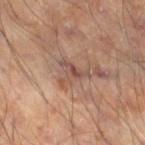Imaged during a routine full-body skin examination; the lesion was not biopsied and no histopathology is available.
About 3.5 mm across.
The lesion is located on the right thigh.
The patient is a male aged 53–57.
A close-up tile cropped from a whole-body skin photograph, about 15 mm across.
Captured under cross-polarized illumination.
An algorithmic analysis of the crop reported a mean CIELAB color near L≈46 a*≈18 b*≈24, roughly 8 lightness units darker than nearby skin, and a normalized lesion–skin contrast near 6. And it measured a border-irregularity rating of about 8/10, a color-variation rating of about 4.5/10, and radial color variation of about 1.5. The software also gave a nevus-likeness score of about 0/100 and a detector confidence of about 65 out of 100 that the crop contains a lesion.